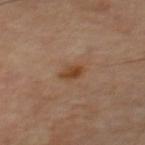No biopsy was performed on this lesion — it was imaged during a full skin examination and was not determined to be concerning.
Located on the upper back.
The patient is a male aged around 60.
A 15 mm close-up extracted from a 3D total-body photography capture.